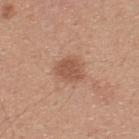Impression:
Imaged during a routine full-body skin examination; the lesion was not biopsied and no histopathology is available.
Acquisition and patient details:
A male patient, in their 30s. The lesion's longest dimension is about 3 mm. The lesion-visualizer software estimated lesion-presence confidence of about 100/100. The lesion is on the upper back. A 15 mm close-up tile from a total-body photography series done for melanoma screening. Captured under white-light illumination.